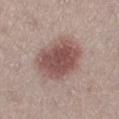The lesion was tiled from a total-body skin photograph and was not biopsied. Captured under white-light illumination. Approximately 6 mm at its widest. A 15 mm close-up extracted from a 3D total-body photography capture. On the left lower leg. The subject is a female aged approximately 25.